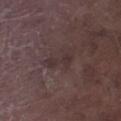workup: catalogued during a skin exam; not biopsied
patient: male, in their mid- to late 70s
lighting: white-light
image: ~15 mm crop, total-body skin-cancer survey
image-analysis metrics: an area of roughly 4 mm², an eccentricity of roughly 0.9, and two-axis asymmetry of about 0.4
body site: the left lower leg